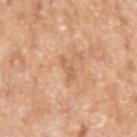follow-up: imaged on a skin check; not biopsied
size: about 2.5 mm
patient: female, roughly 75 years of age
automated metrics: an area of roughly 2.5 mm² and a shape eccentricity near 0.9; a mean CIELAB color near L≈62 a*≈22 b*≈35 and about 7 CIELAB-L* units darker than the surrounding skin
tile lighting: white-light
body site: the left upper arm
image: ~15 mm tile from a whole-body skin photo